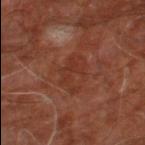| feature | finding |
|---|---|
| image | 15 mm crop, total-body photography |
| subject | male, approximately 60 years of age |
| body site | the right leg |